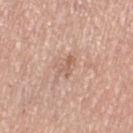Assessment:
No biopsy was performed on this lesion — it was imaged during a full skin examination and was not determined to be concerning.
Acquisition and patient details:
Longest diameter approximately 3 mm. On the right thigh. This image is a 15 mm lesion crop taken from a total-body photograph. Automated tile analysis of the lesion measured a footprint of about 3 mm², a shape eccentricity near 0.85, and two-axis asymmetry of about 0.5. It also reported a lesion–skin lightness drop of about 8. The software also gave a border-irregularity rating of about 6/10, internal color variation of about 0 on a 0–10 scale, and a peripheral color-asymmetry measure near 0. The tile uses white-light illumination. A female patient, in their mid-50s.